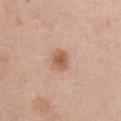Imaged during a routine full-body skin examination; the lesion was not biopsied and no histopathology is available.